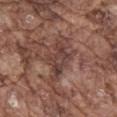The lesion was photographed on a routine skin check and not biopsied; there is no pathology result.